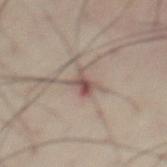– notes · imaged on a skin check; not biopsied
– automated metrics · a lesion area of about 4.5 mm², an eccentricity of roughly 0.75, and a symmetry-axis asymmetry near 0.35; a normalized lesion–skin contrast near 7.5
– image source · total-body-photography crop, ~15 mm field of view
– subject · male, about 55 years old
– tile lighting · cross-polarized illumination
– lesion diameter · about 3.5 mm
– anatomic site · the abdomen